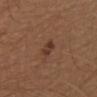biopsy status: imaged on a skin check; not biopsied | image source: ~15 mm crop, total-body skin-cancer survey | size: ~2.5 mm (longest diameter) | subject: female, aged 38–42 | illumination: white-light illumination | site: the upper back.